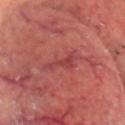Q: Was a biopsy performed?
A: catalogued during a skin exam; not biopsied
Q: What kind of image is this?
A: 15 mm crop, total-body photography
Q: How was the tile lit?
A: cross-polarized
Q: What is the anatomic site?
A: the head or neck
Q: Patient demographics?
A: male, about 65 years old